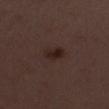{"biopsy_status": "not biopsied; imaged during a skin examination", "lighting": "white-light", "automated_metrics": {"area_mm2_approx": 5.0, "eccentricity": 0.75, "shape_asymmetry": 0.2, "nevus_likeness_0_100": 85}, "patient": {"sex": "female", "age_approx": 50}, "site": "left lower leg", "lesion_size": {"long_diameter_mm_approx": 3.0}, "image": {"source": "total-body photography crop", "field_of_view_mm": 15}}